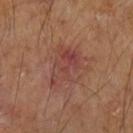Clinical impression:
The lesion was tiled from a total-body skin photograph and was not biopsied.
Background:
The lesion is located on the left forearm. Measured at roughly 5 mm in maximum diameter. Cropped from a total-body skin-imaging series; the visible field is about 15 mm. The tile uses cross-polarized illumination. An algorithmic analysis of the crop reported a footprint of about 14 mm², a shape eccentricity near 0.65, and a symmetry-axis asymmetry near 0.4. It also reported a normalized border contrast of about 6. The software also gave a border-irregularity index near 5/10, a color-variation rating of about 6/10, and radial color variation of about 1.5.